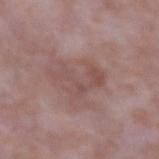<record>
<site>left forearm</site>
<image>
  <source>total-body photography crop</source>
  <field_of_view_mm>15</field_of_view_mm>
</image>
<patient>
  <sex>male</sex>
  <age_approx>65</age_approx>
</patient>
<lighting>white-light</lighting>
<automated_metrics>
  <cielab_L>50</cielab_L>
  <cielab_a>19</cielab_a>
  <cielab_b>21</cielab_b>
  <vs_skin_darker_L>7.0</vs_skin_darker_L>
  <vs_skin_contrast_norm>5.5</vs_skin_contrast_norm>
</automated_metrics>
<lesion_size>
  <long_diameter_mm_approx>5.5</long_diameter_mm_approx>
</lesion_size>
</record>